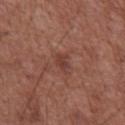Q: Was this lesion biopsied?
A: total-body-photography surveillance lesion; no biopsy
Q: How was the tile lit?
A: white-light
Q: What is the imaging modality?
A: total-body-photography crop, ~15 mm field of view
Q: Where on the body is the lesion?
A: the chest
Q: Patient demographics?
A: male, in their mid-70s
Q: How large is the lesion?
A: ≈2.5 mm